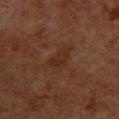No biopsy was performed on this lesion — it was imaged during a full skin examination and was not determined to be concerning. Longest diameter approximately 3.5 mm. Cropped from a whole-body photographic skin survey; the tile spans about 15 mm. The subject is a male aged 58 to 62. Located on the chest. This is a cross-polarized tile.